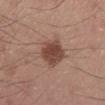Imaged during a routine full-body skin examination; the lesion was not biopsied and no histopathology is available.